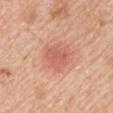Clinical impression: The lesion was photographed on a routine skin check and not biopsied; there is no pathology result. Clinical summary: A close-up tile cropped from a whole-body skin photograph, about 15 mm across. This is a white-light tile. A male subject roughly 50 years of age. Located on the right upper arm.